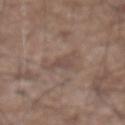{"biopsy_status": "not biopsied; imaged during a skin examination", "patient": {"sex": "male", "age_approx": 75}, "site": "abdomen", "lighting": "white-light", "image": {"source": "total-body photography crop", "field_of_view_mm": 15}, "lesion_size": {"long_diameter_mm_approx": 4.0}}